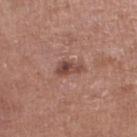Imaged during a routine full-body skin examination; the lesion was not biopsied and no histopathology is available.
The lesion is on the leg.
The tile uses white-light illumination.
A female patient, approximately 70 years of age.
The lesion's longest dimension is about 3 mm.
An algorithmic analysis of the crop reported about 11 CIELAB-L* units darker than the surrounding skin and a lesion-to-skin contrast of about 8 (normalized; higher = more distinct). It also reported a classifier nevus-likeness of about 50/100 and a lesion-detection confidence of about 100/100.
A 15 mm crop from a total-body photograph taken for skin-cancer surveillance.